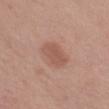biopsy_status: not biopsied; imaged during a skin examination